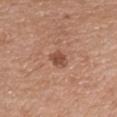Captured during whole-body skin photography for melanoma surveillance; the lesion was not biopsied. Cropped from a total-body skin-imaging series; the visible field is about 15 mm. A male subject in their mid- to late 70s. Captured under white-light illumination. From the chest. Approximately 2.5 mm at its widest.